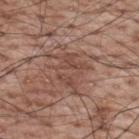The lesion was tiled from a total-body skin photograph and was not biopsied. A male patient aged approximately 70. On the back. Cropped from a whole-body photographic skin survey; the tile spans about 15 mm. Automated tile analysis of the lesion measured a lesion area of about 11 mm², a shape eccentricity near 0.65, and a symmetry-axis asymmetry near 0.5. It also reported a border-irregularity rating of about 7.5/10 and internal color variation of about 4 on a 0–10 scale. It also reported an automated nevus-likeness rating near 0 out of 100 and a detector confidence of about 75 out of 100 that the crop contains a lesion. The recorded lesion diameter is about 5.5 mm.